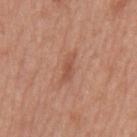Recorded during total-body skin imaging; not selected for excision or biopsy. The lesion's longest dimension is about 3.5 mm. Cropped from a whole-body photographic skin survey; the tile spans about 15 mm. The lesion is located on the mid back. Captured under white-light illumination. The subject is a male aged 68 to 72.